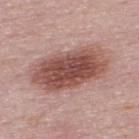{
  "biopsy_status": "not biopsied; imaged during a skin examination",
  "lighting": "white-light",
  "automated_metrics": {
    "color_variation_0_10": 5.5,
    "peripheral_color_asymmetry": 1.5,
    "lesion_detection_confidence_0_100": 100
  },
  "patient": {
    "sex": "male",
    "age_approx": 50
  },
  "lesion_size": {
    "long_diameter_mm_approx": 8.5
  },
  "site": "upper back",
  "image": {
    "source": "total-body photography crop",
    "field_of_view_mm": 15
  }
}